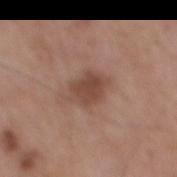<record>
<biopsy_status>not biopsied; imaged during a skin examination</biopsy_status>
<lighting>white-light</lighting>
<lesion_size>
  <long_diameter_mm_approx>4.0</long_diameter_mm_approx>
</lesion_size>
<site>mid back</site>
<image>
  <source>total-body photography crop</source>
  <field_of_view_mm>15</field_of_view_mm>
</image>
<automated_metrics>
  <cielab_L>46</cielab_L>
  <cielab_a>20</cielab_a>
  <cielab_b>26</cielab_b>
  <vs_skin_darker_L>10.0</vs_skin_darker_L>
  <vs_skin_contrast_norm>7.5</vs_skin_contrast_norm>
  <nevus_likeness_0_100>60</nevus_likeness_0_100>
  <lesion_detection_confidence_0_100>100</lesion_detection_confidence_0_100>
</automated_metrics>
<patient>
  <sex>male</sex>
  <age_approx>55</age_approx>
</patient>
</record>